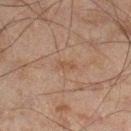follow-up: imaged on a skin check; not biopsied | illumination: cross-polarized | lesion size: ~2.5 mm (longest diameter) | patient: male, aged 43–47 | location: the right lower leg | image source: ~15 mm crop, total-body skin-cancer survey | image-analysis metrics: a border-irregularity index near 3.5/10 and peripheral color asymmetry of about 0; a lesion-detection confidence of about 100/100.